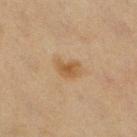body site: the right lower leg | illumination: cross-polarized | image-analysis metrics: an area of roughly 5.5 mm² and an outline eccentricity of about 0.75 (0 = round, 1 = elongated); an average lesion color of about L≈47 a*≈15 b*≈33 (CIELAB), roughly 7 lightness units darker than nearby skin, and a lesion-to-skin contrast of about 7 (normalized; higher = more distinct); a border-irregularity rating of about 3/10 and a within-lesion color-variation index near 2.5/10; an automated nevus-likeness rating near 65 out of 100 and lesion-presence confidence of about 100/100 | subject: female, aged 38–42 | acquisition: total-body-photography crop, ~15 mm field of view | size: ~3 mm (longest diameter).